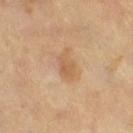workup=imaged on a skin check; not biopsied
image=~15 mm tile from a whole-body skin photo
lesion diameter=≈2.5 mm
patient=male, about 65 years old
illumination=cross-polarized illumination
location=the leg